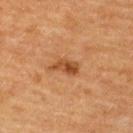No biopsy was performed on this lesion — it was imaged during a full skin examination and was not determined to be concerning. A female patient, approximately 55 years of age. This image is a 15 mm lesion crop taken from a total-body photograph. The total-body-photography lesion software estimated a lesion area of about 4.5 mm², a shape eccentricity near 0.85, and two-axis asymmetry of about 0.35. The analysis additionally found about 10 CIELAB-L* units darker than the surrounding skin and a normalized border contrast of about 8. And it measured a border-irregularity rating of about 3.5/10 and internal color variation of about 4.5 on a 0–10 scale. The analysis additionally found a lesion-detection confidence of about 100/100. Imaged with cross-polarized lighting. The lesion's longest dimension is about 3.5 mm. On the upper back.